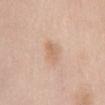Located on the front of the torso.
Cropped from a whole-body photographic skin survey; the tile spans about 15 mm.
A female patient in their 50s.